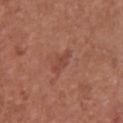<lesion>
  <biopsy_status>not biopsied; imaged during a skin examination</biopsy_status>
  <patient>
    <sex>male</sex>
    <age_approx>65</age_approx>
  </patient>
  <image>
    <source>total-body photography crop</source>
    <field_of_view_mm>15</field_of_view_mm>
  </image>
  <lesion_size>
    <long_diameter_mm_approx>3.5</long_diameter_mm_approx>
  </lesion_size>
  <site>chest</site>
</lesion>